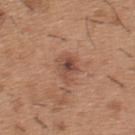Clinical impression:
This lesion was catalogued during total-body skin photography and was not selected for biopsy.
Image and clinical context:
Automated tile analysis of the lesion measured a lesion color around L≈49 a*≈21 b*≈29 in CIELAB, a lesion–skin lightness drop of about 9, and a normalized lesion–skin contrast near 7. The analysis additionally found a classifier nevus-likeness of about 25/100 and lesion-presence confidence of about 100/100. The tile uses white-light illumination. A male subject aged 38–42. On the upper back. A 15 mm crop from a total-body photograph taken for skin-cancer surveillance.